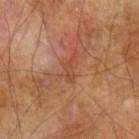biopsy_status: not biopsied; imaged during a skin examination
image:
  source: total-body photography crop
  field_of_view_mm: 15
patient:
  sex: male
  age_approx: 70
site: left forearm
lesion_size:
  long_diameter_mm_approx: 3.0